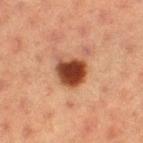Captured during whole-body skin photography for melanoma surveillance; the lesion was not biopsied. Longest diameter approximately 3.5 mm. A 15 mm crop from a total-body photograph taken for skin-cancer surveillance. The lesion is on the left thigh. The lesion-visualizer software estimated a border-irregularity index near 2/10, internal color variation of about 4 on a 0–10 scale, and peripheral color asymmetry of about 1. A female patient aged around 55.